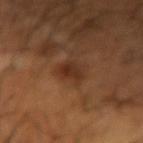Q: Is there a histopathology result?
A: no biopsy performed (imaged during a skin exam)
Q: Automated lesion metrics?
A: a lesion area of about 5.5 mm²; an average lesion color of about L≈32 a*≈21 b*≈29 (CIELAB) and a normalized border contrast of about 8
Q: Illumination type?
A: cross-polarized illumination
Q: What is the imaging modality?
A: ~15 mm tile from a whole-body skin photo
Q: Patient demographics?
A: male, in their mid-60s
Q: What is the lesion's diameter?
A: about 3.5 mm
Q: What is the anatomic site?
A: the left forearm